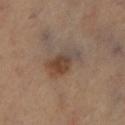| field | value |
|---|---|
| follow-up | no biopsy performed (imaged during a skin exam) |
| patient | female, aged 68–72 |
| anatomic site | the left lower leg |
| image source | 15 mm crop, total-body photography |